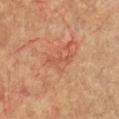TBP lesion metrics = border irregularity of about 7 on a 0–10 scale and internal color variation of about 0 on a 0–10 scale; a nevus-likeness score of about 40/100 and lesion-presence confidence of about 100/100 | imaging modality = ~15 mm tile from a whole-body skin photo | patient = female, aged 78 to 82 | body site = the chest | size = ~3.5 mm (longest diameter) | tile lighting = cross-polarized.